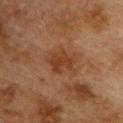Notes:
* notes · no biopsy performed (imaged during a skin exam)
* image source · ~15 mm tile from a whole-body skin photo
* subject · male, aged approximately 75
* lighting · cross-polarized illumination
* lesion size · about 4 mm
* automated lesion analysis · a mean CIELAB color near L≈32 a*≈20 b*≈28, about 7 CIELAB-L* units darker than the surrounding skin, and a lesion-to-skin contrast of about 7 (normalized; higher = more distinct); a nevus-likeness score of about 0/100 and lesion-presence confidence of about 100/100
* location · the chest